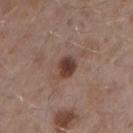Imaged during a routine full-body skin examination; the lesion was not biopsied and no histopathology is available.
A roughly 15 mm field-of-view crop from a total-body skin photograph.
Automated image analysis of the tile measured a footprint of about 5 mm² and a symmetry-axis asymmetry near 0.2. And it measured an average lesion color of about L≈39 a*≈19 b*≈23 (CIELAB) and a normalized lesion–skin contrast near 10. It also reported a border-irregularity index near 2/10 and a peripheral color-asymmetry measure near 1. The analysis additionally found a nevus-likeness score of about 90/100 and a lesion-detection confidence of about 100/100.
On the right upper arm.
Measured at roughly 2.5 mm in maximum diameter.
The subject is a male aged approximately 55.
Captured under white-light illumination.